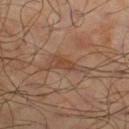This lesion was catalogued during total-body skin photography and was not selected for biopsy. The tile uses cross-polarized illumination. From the right lower leg. A region of skin cropped from a whole-body photographic capture, roughly 15 mm wide. A male patient, aged 63–67. Longest diameter approximately 5 mm.